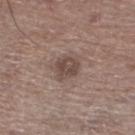follow-up: catalogued during a skin exam; not biopsied | imaging modality: total-body-photography crop, ~15 mm field of view | body site: the left lower leg | lesion size: ~3 mm (longest diameter) | subject: male, in their mid-60s | lighting: white-light illumination.